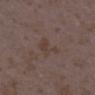biopsy_status: not biopsied; imaged during a skin examination
patient:
  sex: female
  age_approx: 35
site: right lower leg
lesion_size:
  long_diameter_mm_approx: 3.0
image:
  source: total-body photography crop
  field_of_view_mm: 15